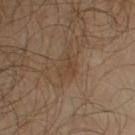{"biopsy_status": "not biopsied; imaged during a skin examination", "image": {"source": "total-body photography crop", "field_of_view_mm": 15}, "site": "right upper arm", "automated_metrics": {"area_mm2_approx": 5.0, "eccentricity": 0.75, "cielab_L": 38, "cielab_a": 14, "cielab_b": 27, "vs_skin_contrast_norm": 5.0, "border_irregularity_0_10": 4.5, "color_variation_0_10": 2.0, "peripheral_color_asymmetry": 0.5, "nevus_likeness_0_100": 0, "lesion_detection_confidence_0_100": 100}, "patient": {"sex": "male", "age_approx": 65}, "lighting": "cross-polarized"}